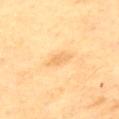workup: catalogued during a skin exam; not biopsied
subject: male, aged approximately 55
automated metrics: a lesion color around L≈71 a*≈18 b*≈44 in CIELAB, roughly 7 lightness units darker than nearby skin, and a lesion-to-skin contrast of about 4.5 (normalized; higher = more distinct); border irregularity of about 2.5 on a 0–10 scale, a color-variation rating of about 1/10, and a peripheral color-asymmetry measure near 0.5
location: the upper back
lesion diameter: ~3 mm (longest diameter)
acquisition: 15 mm crop, total-body photography